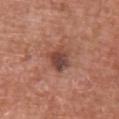subject: male, aged 43 to 47 | anatomic site: the upper back | imaging modality: ~15 mm crop, total-body skin-cancer survey.